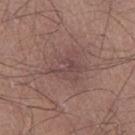follow-up: imaged on a skin check; not biopsied
tile lighting: white-light
patient: male, in their mid-20s
site: the left thigh
TBP lesion metrics: a shape-asymmetry score of about 0.4 (0 = symmetric); an average lesion color of about L≈45 a*≈18 b*≈19 (CIELAB), about 6 CIELAB-L* units darker than the surrounding skin, and a normalized lesion–skin contrast near 5; border irregularity of about 4.5 on a 0–10 scale and a color-variation rating of about 4/10
diameter: ≈4.5 mm
acquisition: total-body-photography crop, ~15 mm field of view